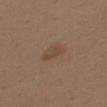Part of a total-body skin-imaging series; this lesion was reviewed on a skin check and was not flagged for biopsy.
On the upper back.
A 15 mm close-up tile from a total-body photography series done for melanoma screening.
A male patient in their 40s.